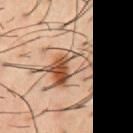- workup · catalogued during a skin exam; not biopsied
- lesion diameter · ≈7.5 mm
- site · the chest
- subject · male, in their mid- to late 50s
- image source · ~15 mm crop, total-body skin-cancer survey
- illumination · cross-polarized
- automated metrics · a lesion area of about 19 mm², a shape eccentricity near 0.85, and a shape-asymmetry score of about 0.5 (0 = symmetric); a lesion–skin lightness drop of about 12 and a lesion-to-skin contrast of about 8 (normalized; higher = more distinct); border irregularity of about 7.5 on a 0–10 scale, a color-variation rating of about 10/10, and peripheral color asymmetry of about 6.5